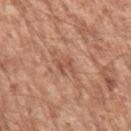Captured during whole-body skin photography for melanoma surveillance; the lesion was not biopsied. The lesion is on the left upper arm. The patient is a male aged 63–67. Longest diameter approximately 2.5 mm. Automated image analysis of the tile measured an area of roughly 3.5 mm² and a symmetry-axis asymmetry near 0.35. And it measured a classifier nevus-likeness of about 0/100 and a lesion-detection confidence of about 95/100. The tile uses white-light illumination. This image is a 15 mm lesion crop taken from a total-body photograph.